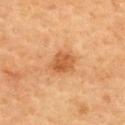Case summary:
• follow-up — total-body-photography surveillance lesion; no biopsy
• body site — the upper back
• automated lesion analysis — an area of roughly 6.5 mm², a shape eccentricity near 0.6, and a shape-asymmetry score of about 0.2 (0 = symmetric); a lesion color around L≈51 a*≈24 b*≈39 in CIELAB; an automated nevus-likeness rating near 70 out of 100
• imaging modality — ~15 mm tile from a whole-body skin photo
• lesion size — ~3.5 mm (longest diameter)
• subject — female, aged 58–62